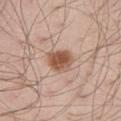Q: Who is the patient?
A: male, in their mid- to late 50s
Q: Lesion location?
A: the right thigh
Q: What kind of image is this?
A: 15 mm crop, total-body photography